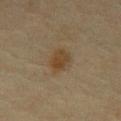follow-up = imaged on a skin check; not biopsied | subject = male, about 70 years old | image = ~15 mm crop, total-body skin-cancer survey | body site = the back | size = ≈3.5 mm | illumination = cross-polarized illumination.